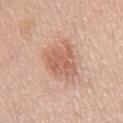lighting: white-light illumination
image source: total-body-photography crop, ~15 mm field of view
patient: female, aged 63 to 67
body site: the chest